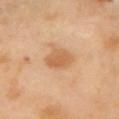A female patient, in their mid-50s.
Longest diameter approximately 3.5 mm.
A roughly 15 mm field-of-view crop from a total-body skin photograph.
The lesion is located on the left upper arm.